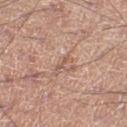{
  "biopsy_status": "not biopsied; imaged during a skin examination",
  "site": "left thigh",
  "lighting": "white-light",
  "patient": {
    "sex": "male",
    "age_approx": 45
  },
  "image": {
    "source": "total-body photography crop",
    "field_of_view_mm": 15
  }
}